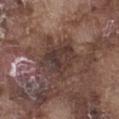A male patient approximately 75 years of age. Cropped from a total-body skin-imaging series; the visible field is about 15 mm. The total-body-photography lesion software estimated an area of roughly 18 mm², an eccentricity of roughly 0.55, and a shape-asymmetry score of about 0.25 (0 = symmetric). The software also gave a lesion color around L≈37 a*≈16 b*≈20 in CIELAB, roughly 8 lightness units darker than nearby skin, and a normalized border contrast of about 7.5. The software also gave border irregularity of about 4.5 on a 0–10 scale, a color-variation rating of about 5.5/10, and a peripheral color-asymmetry measure near 1.5. Imaged with white-light lighting. From the leg. Measured at roughly 5.5 mm in maximum diameter.